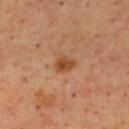Q: Was a biopsy performed?
A: imaged on a skin check; not biopsied
Q: Illumination type?
A: cross-polarized illumination
Q: What are the patient's age and sex?
A: male, in their mid- to late 60s
Q: What is the anatomic site?
A: the upper back
Q: How was this image acquired?
A: ~15 mm crop, total-body skin-cancer survey
Q: How large is the lesion?
A: ~2.5 mm (longest diameter)